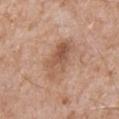A male patient, aged 63 to 67.
This image is a 15 mm lesion crop taken from a total-body photograph.
About 5.5 mm across.
The lesion is located on the chest.